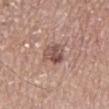The lesion was photographed on a routine skin check and not biopsied; there is no pathology result.
A male subject aged 73–77.
Cropped from a whole-body photographic skin survey; the tile spans about 15 mm.
From the leg.